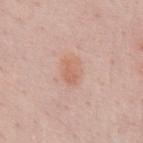<tbp_lesion>
  <biopsy_status>not biopsied; imaged during a skin examination</biopsy_status>
  <image>
    <source>total-body photography crop</source>
    <field_of_view_mm>15</field_of_view_mm>
  </image>
  <patient>
    <sex>male</sex>
    <age_approx>55</age_approx>
  </patient>
  <lighting>white-light</lighting>
  <site>chest</site>
  <lesion_size>
    <long_diameter_mm_approx>3.0</long_diameter_mm_approx>
  </lesion_size>
</tbp_lesion>